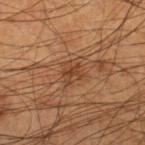{"biopsy_status": "not biopsied; imaged during a skin examination", "patient": {"sex": "male", "age_approx": 55}, "site": "right lower leg", "automated_metrics": {"area_mm2_approx": 5.5, "eccentricity": 0.4, "shape_asymmetry": 0.35, "cielab_L": 39, "cielab_a": 20, "cielab_b": 31, "vs_skin_darker_L": 7.0, "vs_skin_contrast_norm": 6.5, "border_irregularity_0_10": 4.0, "peripheral_color_asymmetry": 1.0}, "lesion_size": {"long_diameter_mm_approx": 3.0}, "image": {"source": "total-body photography crop", "field_of_view_mm": 15}, "lighting": "cross-polarized"}